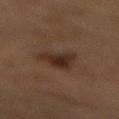Imaged during a routine full-body skin examination; the lesion was not biopsied and no histopathology is available. Located on the back. This image is a 15 mm lesion crop taken from a total-body photograph. About 4 mm across. The lesion-visualizer software estimated a mean CIELAB color near L≈24 a*≈14 b*≈21, a lesion–skin lightness drop of about 8, and a normalized border contrast of about 9.5. A male subject roughly 85 years of age or older.